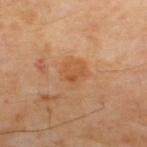biopsy status: total-body-photography surveillance lesion; no biopsy
imaging modality: ~15 mm crop, total-body skin-cancer survey
TBP lesion metrics: an area of roughly 7.5 mm², an outline eccentricity of about 0.35 (0 = round, 1 = elongated), and two-axis asymmetry of about 0.25; a mean CIELAB color near L≈52 a*≈23 b*≈37; lesion-presence confidence of about 100/100
patient: male, about 65 years old
illumination: cross-polarized
diameter: ~3.5 mm (longest diameter)
location: the upper back